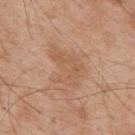Impression:
Recorded during total-body skin imaging; not selected for excision or biopsy.
Context:
Cropped from a whole-body photographic skin survey; the tile spans about 15 mm. Captured under white-light illumination. The lesion is on the mid back. About 5 mm across. A male patient aged around 55.